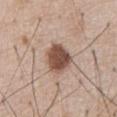The lesion was photographed on a routine skin check and not biopsied; there is no pathology result. The lesion is located on the front of the torso. A lesion tile, about 15 mm wide, cut from a 3D total-body photograph. This is a white-light tile. Measured at roughly 4 mm in maximum diameter. Automated tile analysis of the lesion measured a border-irregularity index near 1.5/10 and a peripheral color-asymmetry measure near 1. A male subject roughly 60 years of age.